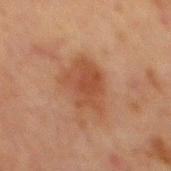{
  "biopsy_status": "not biopsied; imaged during a skin examination"
}